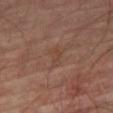The lesion was photographed on a routine skin check and not biopsied; there is no pathology result.
The lesion's longest dimension is about 3 mm.
A 15 mm close-up extracted from a 3D total-body photography capture.
The tile uses cross-polarized illumination.
A male patient aged approximately 70.
The lesion is located on the leg.